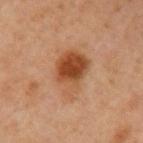{"biopsy_status": "not biopsied; imaged during a skin examination", "lesion_size": {"long_diameter_mm_approx": 5.5}, "image": {"source": "total-body photography crop", "field_of_view_mm": 15}, "automated_metrics": {"shape_asymmetry": 0.3, "border_irregularity_0_10": 3.5, "color_variation_0_10": 7.0, "peripheral_color_asymmetry": 2.0, "nevus_likeness_0_100": 100}, "site": "left upper arm", "patient": {"sex": "male", "age_approx": 45}, "lighting": "cross-polarized"}